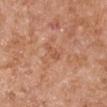Part of a total-body skin-imaging series; this lesion was reviewed on a skin check and was not flagged for biopsy.
The tile uses white-light illumination.
The recorded lesion diameter is about 2.5 mm.
A male patient, roughly 65 years of age.
On the right upper arm.
Cropped from a whole-body photographic skin survey; the tile spans about 15 mm.